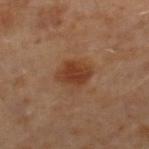Automated image analysis of the tile measured roughly 9 lightness units darker than nearby skin and a normalized border contrast of about 8.5. The analysis additionally found a classifier nevus-likeness of about 95/100.
Cropped from a total-body skin-imaging series; the visible field is about 15 mm.
The lesion's longest dimension is about 3.5 mm.
A male subject, aged 58 to 62.
The lesion is on the right lower leg.
The tile uses cross-polarized illumination.